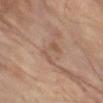Case summary:
- anatomic site: the right leg
- tile lighting: cross-polarized illumination
- size: ~3.5 mm (longest diameter)
- imaging modality: ~15 mm crop, total-body skin-cancer survey
- patient: female, about 80 years old